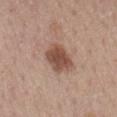Impression: Captured during whole-body skin photography for melanoma surveillance; the lesion was not biopsied. Context: This is a white-light tile. About 4 mm across. A lesion tile, about 15 mm wide, cut from a 3D total-body photograph. From the mid back. A female patient, roughly 65 years of age.